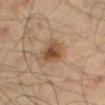notes: imaged on a skin check; not biopsied
subject: male, roughly 70 years of age
image: total-body-photography crop, ~15 mm field of view
size: about 3.5 mm
illumination: cross-polarized illumination
anatomic site: the right thigh